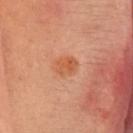follow-up = imaged on a skin check; not biopsied | image source = 15 mm crop, total-body photography | diameter = ≈3 mm | illumination = cross-polarized illumination | patient = female, aged around 40 | anatomic site = the head or neck.